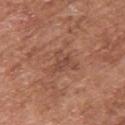• notes · catalogued during a skin exam; not biopsied
• size · ≈3.5 mm
• lighting · white-light
• image · 15 mm crop, total-body photography
• subject · male, aged around 75
• location · the upper back